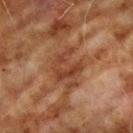No biopsy was performed on this lesion — it was imaged during a full skin examination and was not determined to be concerning. The lesion is located on the right upper arm. A region of skin cropped from a whole-body photographic capture, roughly 15 mm wide. The lesion-visualizer software estimated a lesion color around L≈34 a*≈21 b*≈27 in CIELAB, roughly 7 lightness units darker than nearby skin, and a normalized lesion–skin contrast near 6.5. The software also gave a nevus-likeness score of about 0/100 and a detector confidence of about 80 out of 100 that the crop contains a lesion. About 4 mm across. Captured under cross-polarized illumination. The subject is a male aged 68 to 72.